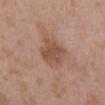notes = total-body-photography surveillance lesion; no biopsy
acquisition = ~15 mm tile from a whole-body skin photo
subject = female, aged 33–37
anatomic site = the right upper arm